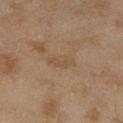Impression: Captured during whole-body skin photography for melanoma surveillance; the lesion was not biopsied. Background: The subject is a female in their 60s. This image is a 15 mm lesion crop taken from a total-body photograph. The lesion is located on the right lower leg.